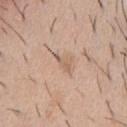Captured during whole-body skin photography for melanoma surveillance; the lesion was not biopsied. A male subject roughly 40 years of age. On the chest. Imaged with white-light lighting. Cropped from a total-body skin-imaging series; the visible field is about 15 mm. Automated tile analysis of the lesion measured a border-irregularity rating of about 5.5/10 and a color-variation rating of about 0/10. Longest diameter approximately 3 mm.